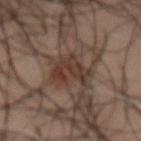workup: no biopsy performed (imaged during a skin exam) | automated metrics: a lesion color around L≈34 a*≈16 b*≈22 in CIELAB, roughly 9 lightness units darker than nearby skin, and a lesion-to-skin contrast of about 8.5 (normalized; higher = more distinct); a lesion-detection confidence of about 75/100 | body site: the front of the torso | size: ~4.5 mm (longest diameter) | lighting: cross-polarized | acquisition: ~15 mm crop, total-body skin-cancer survey | patient: male, about 65 years old.